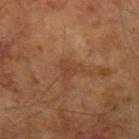Assessment:
Captured during whole-body skin photography for melanoma surveillance; the lesion was not biopsied.
Acquisition and patient details:
A roughly 15 mm field-of-view crop from a total-body skin photograph. From the left upper arm. The patient is a male roughly 65 years of age.